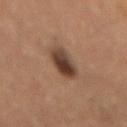Q: Was a biopsy performed?
A: total-body-photography surveillance lesion; no biopsy
Q: What lighting was used for the tile?
A: cross-polarized
Q: Lesion size?
A: about 4.5 mm
Q: What kind of image is this?
A: ~15 mm tile from a whole-body skin photo
Q: What did automated image analysis measure?
A: a lesion color around L≈34 a*≈16 b*≈23 in CIELAB, roughly 13 lightness units darker than nearby skin, and a normalized lesion–skin contrast near 11.5; an automated nevus-likeness rating near 95 out of 100 and a lesion-detection confidence of about 100/100
Q: Who is the patient?
A: male, roughly 60 years of age